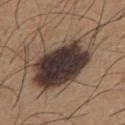Clinical impression: Imaged during a routine full-body skin examination; the lesion was not biopsied and no histopathology is available. Image and clinical context: The lesion-visualizer software estimated a border-irregularity index near 4.5/10 and a within-lesion color-variation index near 9.5/10. The recorded lesion diameter is about 10.5 mm. The lesion is on the chest. A male subject aged 63 to 67. A 15 mm close-up extracted from a 3D total-body photography capture. Imaged with white-light lighting.